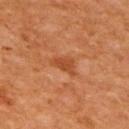Part of a total-body skin-imaging series; this lesion was reviewed on a skin check and was not flagged for biopsy.
This is a cross-polarized tile.
A male subject, about 65 years old.
On the back.
This image is a 15 mm lesion crop taken from a total-body photograph.
An algorithmic analysis of the crop reported an area of roughly 5 mm². The software also gave border irregularity of about 4.5 on a 0–10 scale, a color-variation rating of about 1.5/10, and peripheral color asymmetry of about 0.5.
The recorded lesion diameter is about 3.5 mm.